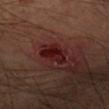Acquisition and patient details:
A male patient aged approximately 45. On the right forearm. A 15 mm close-up extracted from a 3D total-body photography capture.